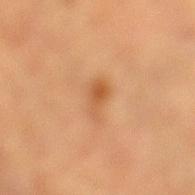A male patient in their mid- to late 80s or older.
Approximately 3 mm at its widest.
The lesion is on the left lower leg.
A close-up tile cropped from a whole-body skin photograph, about 15 mm across.
Imaged with cross-polarized lighting.